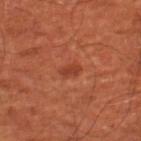workup=imaged on a skin check; not biopsied | patient=aged approximately 65 | size=about 2.5 mm | TBP lesion metrics=a mean CIELAB color near L≈41 a*≈30 b*≈35 and about 8 CIELAB-L* units darker than the surrounding skin | imaging modality=~15 mm tile from a whole-body skin photo | tile lighting=cross-polarized | anatomic site=the right lower leg.